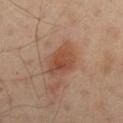{"biopsy_status": "not biopsied; imaged during a skin examination", "image": {"source": "total-body photography crop", "field_of_view_mm": 15}, "site": "left upper arm", "lesion_size": {"long_diameter_mm_approx": 4.5}, "lighting": "cross-polarized", "automated_metrics": {"area_mm2_approx": 11.0, "eccentricity": 0.75, "shape_asymmetry": 0.2}, "patient": {"sex": "male", "age_approx": 50}}